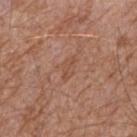follow-up = no biopsy performed (imaged during a skin exam).